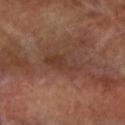Findings:
– follow-up — imaged on a skin check; not biopsied
– site — the left arm
– image source — 15 mm crop, total-body photography
– automated lesion analysis — a footprint of about 8.5 mm², an outline eccentricity of about 0.85 (0 = round, 1 = elongated), and a shape-asymmetry score of about 0.3 (0 = symmetric)
– tile lighting — cross-polarized
– diameter — ≈5 mm
– patient — female, aged around 60